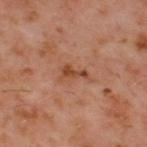This lesion was catalogued during total-body skin photography and was not selected for biopsy.
A male subject, approximately 60 years of age.
The tile uses cross-polarized illumination.
A 15 mm crop from a total-body photograph taken for skin-cancer surveillance.
Automated image analysis of the tile measured a footprint of about 4.5 mm², a shape eccentricity near 0.9, and a shape-asymmetry score of about 0.55 (0 = symmetric). And it measured an average lesion color of about L≈43 a*≈23 b*≈31 (CIELAB), a lesion–skin lightness drop of about 8, and a normalized border contrast of about 7. The analysis additionally found a nevus-likeness score of about 0/100 and lesion-presence confidence of about 100/100.
From the upper back.